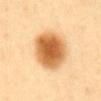| feature | finding |
|---|---|
| patient | female, about 50 years old |
| lesion diameter | about 5.5 mm |
| acquisition | ~15 mm crop, total-body skin-cancer survey |
| anatomic site | the abdomen |
| automated lesion analysis | an average lesion color of about L≈63 a*≈23 b*≈45 (CIELAB), about 18 CIELAB-L* units darker than the surrounding skin, and a lesion-to-skin contrast of about 11 (normalized; higher = more distinct); a nevus-likeness score of about 100/100 and a lesion-detection confidence of about 100/100 |
| lighting | cross-polarized |